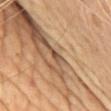Imaged during a routine full-body skin examination; the lesion was not biopsied and no histopathology is available.
The subject is a female in their 60s.
From the front of the torso.
Cropped from a whole-body photographic skin survey; the tile spans about 15 mm.